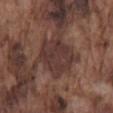<record>
<biopsy_status>not biopsied; imaged during a skin examination</biopsy_status>
<lighting>white-light</lighting>
<lesion_size>
  <long_diameter_mm_approx>5.0</long_diameter_mm_approx>
</lesion_size>
<patient>
  <sex>male</sex>
  <age_approx>75</age_approx>
</patient>
<automated_metrics>
  <area_mm2_approx>14.0</area_mm2_approx>
  <shape_asymmetry>0.2</shape_asymmetry>
</automated_metrics>
<site>mid back</site>
<image>
  <source>total-body photography crop</source>
  <field_of_view_mm>15</field_of_view_mm>
</image>
</record>